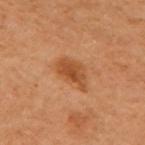A 15 mm close-up extracted from a 3D total-body photography capture.
Located on the chest.
The lesion's longest dimension is about 4 mm.
The subject is a female approximately 60 years of age.
Automated tile analysis of the lesion measured a footprint of about 8.5 mm², an eccentricity of roughly 0.75, and two-axis asymmetry of about 0.35. The analysis additionally found a border-irregularity rating of about 3/10, a within-lesion color-variation index near 3/10, and peripheral color asymmetry of about 1. It also reported a nevus-likeness score of about 90/100 and lesion-presence confidence of about 100/100.
Captured under cross-polarized illumination.